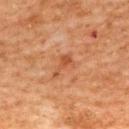This lesion was catalogued during total-body skin photography and was not selected for biopsy. The tile uses cross-polarized illumination. A female subject in their 60s. Approximately 3 mm at its widest. This image is a 15 mm lesion crop taken from a total-body photograph. Automated image analysis of the tile measured a lesion–skin lightness drop of about 7 and a normalized border contrast of about 6. The software also gave a border-irregularity rating of about 7/10, a color-variation rating of about 0.5/10, and a peripheral color-asymmetry measure near 0. And it measured an automated nevus-likeness rating near 0 out of 100. The lesion is located on the upper back.